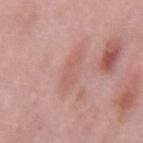Image and clinical context:
Cropped from a total-body skin-imaging series; the visible field is about 15 mm. The lesion is on the mid back. The subject is a male aged 53–57. The lesion's longest dimension is about 4 mm. The tile uses white-light illumination.